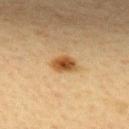The lesion was tiled from a total-body skin photograph and was not biopsied. On the upper back. A female subject, roughly 45 years of age. A 15 mm close-up extracted from a 3D total-body photography capture.